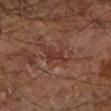body site = the left lower leg; acquisition = ~15 mm crop, total-body skin-cancer survey; patient = aged approximately 65.